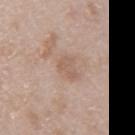Part of a total-body skin-imaging series; this lesion was reviewed on a skin check and was not flagged for biopsy.
The tile uses white-light illumination.
A 15 mm close-up tile from a total-body photography series done for melanoma screening.
The subject is a female aged 48 to 52.
Automated image analysis of the tile measured a mean CIELAB color near L≈58 a*≈17 b*≈28, about 7 CIELAB-L* units darker than the surrounding skin, and a lesion-to-skin contrast of about 5.5 (normalized; higher = more distinct). It also reported a border-irregularity index near 5/10, internal color variation of about 1 on a 0–10 scale, and peripheral color asymmetry of about 0.5.
The lesion is on the right upper arm.